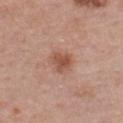<case>
<biopsy_status>not biopsied; imaged during a skin examination</biopsy_status>
<site>chest</site>
<image>
  <source>total-body photography crop</source>
  <field_of_view_mm>15</field_of_view_mm>
</image>
<patient>
  <sex>male</sex>
  <age_approx>60</age_approx>
</patient>
</case>